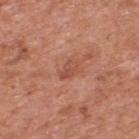Q: Was a biopsy performed?
A: no biopsy performed (imaged during a skin exam)
Q: Illumination type?
A: white-light
Q: What is the anatomic site?
A: the upper back
Q: Patient demographics?
A: male, approximately 70 years of age
Q: What is the imaging modality?
A: 15 mm crop, total-body photography